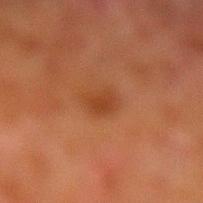Assessment: The lesion was photographed on a routine skin check and not biopsied; there is no pathology result. Context: The lesion is on the left lower leg. About 2.5 mm across. A region of skin cropped from a whole-body photographic capture, roughly 15 mm wide. A male patient aged 78 to 82. Imaged with cross-polarized lighting.